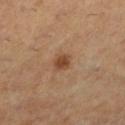Impression:
Imaged during a routine full-body skin examination; the lesion was not biopsied and no histopathology is available.
Clinical summary:
On the leg. A 15 mm close-up tile from a total-body photography series done for melanoma screening. The lesion's longest dimension is about 2 mm. The patient is a female approximately 55 years of age. The total-body-photography lesion software estimated an average lesion color of about L≈45 a*≈21 b*≈34 (CIELAB), roughly 11 lightness units darker than nearby skin, and a normalized border contrast of about 8.5. And it measured a border-irregularity index near 2/10, a within-lesion color-variation index near 1.5/10, and radial color variation of about 0.5.